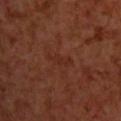Context: This is a cross-polarized tile. The recorded lesion diameter is about 3.5 mm. The subject is a male roughly 70 years of age. The lesion is on the upper back. A close-up tile cropped from a whole-body skin photograph, about 15 mm across.